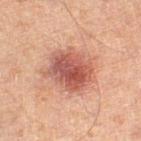follow-up = total-body-photography surveillance lesion; no biopsy | patient = male, about 65 years old | site = the left thigh | diameter = ≈5.5 mm | illumination = cross-polarized illumination | automated lesion analysis = an area of roughly 18 mm² and a shape eccentricity near 0.6; a border-irregularity rating of about 2.5/10, internal color variation of about 6 on a 0–10 scale, and peripheral color asymmetry of about 2; an automated nevus-likeness rating near 70 out of 100 and a detector confidence of about 100 out of 100 that the crop contains a lesion | imaging modality = total-body-photography crop, ~15 mm field of view.